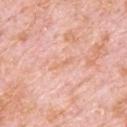Impression:
Captured during whole-body skin photography for melanoma surveillance; the lesion was not biopsied.
Background:
A male patient, aged 78 to 82. A roughly 15 mm field-of-view crop from a total-body skin photograph. The lesion is located on the back. Imaged with white-light lighting. About 3 mm across.